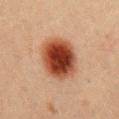<lesion>
  <biopsy_status>not biopsied; imaged during a skin examination</biopsy_status>
  <lighting>cross-polarized</lighting>
  <site>chest</site>
  <patient>
    <sex>male</sex>
    <age_approx>40</age_approx>
  </patient>
  <image>
    <source>total-body photography crop</source>
    <field_of_view_mm>15</field_of_view_mm>
  </image>
</lesion>